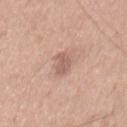workup — catalogued during a skin exam; not biopsied